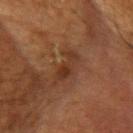Q: Is there a histopathology result?
A: total-body-photography surveillance lesion; no biopsy
Q: Lesion location?
A: the head or neck
Q: Automated lesion metrics?
A: a lesion area of about 5.5 mm² and a symmetry-axis asymmetry near 0.3; border irregularity of about 4 on a 0–10 scale and radial color variation of about 0.5
Q: Illumination type?
A: cross-polarized
Q: Patient demographics?
A: female, approximately 80 years of age
Q: What kind of image is this?
A: ~15 mm crop, total-body skin-cancer survey
Q: What is the lesion's diameter?
A: about 4 mm